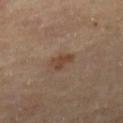Notes:
- notes: no biopsy performed (imaged during a skin exam)
- anatomic site: the right lower leg
- automated lesion analysis: a mean CIELAB color near L≈42 a*≈17 b*≈28, a lesion–skin lightness drop of about 8, and a normalized lesion–skin contrast near 7.5; a border-irregularity index near 4/10, a within-lesion color-variation index near 1/10, and radial color variation of about 0.5; a classifier nevus-likeness of about 15/100 and lesion-presence confidence of about 100/100
- patient: female, about 60 years old
- image source: ~15 mm tile from a whole-body skin photo
- illumination: cross-polarized
- diameter: ≈3 mm